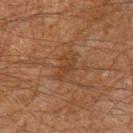Background:
The lesion-visualizer software estimated an eccentricity of roughly 0.85 and a shape-asymmetry score of about 0.45 (0 = symmetric). The analysis additionally found border irregularity of about 5 on a 0–10 scale, internal color variation of about 1 on a 0–10 scale, and a peripheral color-asymmetry measure near 0. The analysis additionally found a nevus-likeness score of about 0/100 and lesion-presence confidence of about 100/100. A lesion tile, about 15 mm wide, cut from a 3D total-body photograph. Measured at roughly 3 mm in maximum diameter. From the right thigh. The subject is a male aged 58 to 62. Imaged with cross-polarized lighting.